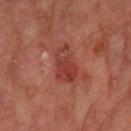{
  "site": "right lower leg",
  "image": {
    "source": "total-body photography crop",
    "field_of_view_mm": 15
  },
  "patient": {
    "sex": "male",
    "age_approx": 70
  },
  "automated_metrics": {
    "border_irregularity_0_10": 3.5,
    "color_variation_0_10": 6.0
  },
  "lesion_size": {
    "long_diameter_mm_approx": 5.0
  }
}